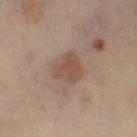Captured during whole-body skin photography for melanoma surveillance; the lesion was not biopsied. A female patient aged around 50. A roughly 15 mm field-of-view crop from a total-body skin photograph. On the right thigh. The tile uses cross-polarized illumination.